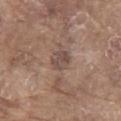Recorded during total-body skin imaging; not selected for excision or biopsy. A 15 mm crop from a total-body photograph taken for skin-cancer surveillance. A male subject, aged 78–82. This is a white-light tile. Measured at roughly 3 mm in maximum diameter. The lesion is on the abdomen.